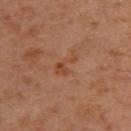automated metrics = a footprint of about 4 mm² and a shape eccentricity near 0.85; an automated nevus-likeness rating near 0 out of 100 | subject = male, in their mid-50s | location = the back | lighting = cross-polarized illumination | image = total-body-photography crop, ~15 mm field of view.